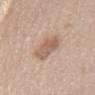The lesion was photographed on a routine skin check and not biopsied; there is no pathology result. A female subject, roughly 75 years of age. Automated tile analysis of the lesion measured an eccentricity of roughly 0.95. It also reported an average lesion color of about L≈60 a*≈18 b*≈27 (CIELAB), roughly 11 lightness units darker than nearby skin, and a normalized lesion–skin contrast near 7. On the mid back. A close-up tile cropped from a whole-body skin photograph, about 15 mm across. This is a white-light tile. About 5.5 mm across.